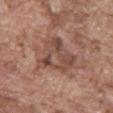Q: Is there a histopathology result?
A: total-body-photography surveillance lesion; no biopsy
Q: Where on the body is the lesion?
A: the abdomen
Q: What kind of image is this?
A: ~15 mm tile from a whole-body skin photo
Q: Patient demographics?
A: male, aged approximately 75